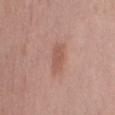Q: Was this lesion biopsied?
A: imaged on a skin check; not biopsied
Q: Illumination type?
A: white-light
Q: Lesion size?
A: ~3.5 mm (longest diameter)
Q: Automated lesion metrics?
A: a lesion color around L≈55 a*≈23 b*≈27 in CIELAB, roughly 8 lightness units darker than nearby skin, and a normalized border contrast of about 6
Q: What is the anatomic site?
A: the leg
Q: How was this image acquired?
A: total-body-photography crop, ~15 mm field of view
Q: Patient demographics?
A: female, about 40 years old